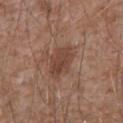Case summary:
– notes — total-body-photography surveillance lesion; no biopsy
– subject — male, aged approximately 60
– imaging modality — ~15 mm crop, total-body skin-cancer survey
– automated metrics — a lesion color around L≈43 a*≈19 b*≈27 in CIELAB and a normalized lesion–skin contrast near 7
– location — the mid back